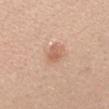Imaged during a routine full-body skin examination; the lesion was not biopsied and no histopathology is available. Captured under white-light illumination. The lesion is on the left forearm. A lesion tile, about 15 mm wide, cut from a 3D total-body photograph. A female patient aged approximately 25. Approximately 2.5 mm at its widest.